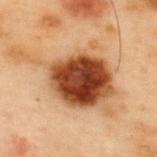Clinical impression:
Imaged during a routine full-body skin examination; the lesion was not biopsied and no histopathology is available.
Image and clinical context:
The lesion is located on the upper back. A 15 mm crop from a total-body photograph taken for skin-cancer surveillance. A male subject aged 53–57. Longest diameter approximately 11.5 mm. Automated image analysis of the tile measured a lesion area of about 38 mm², a shape eccentricity near 0.8, and a shape-asymmetry score of about 0.45 (0 = symmetric). The analysis additionally found a border-irregularity rating of about 6.5/10, a color-variation rating of about 9/10, and a peripheral color-asymmetry measure near 2.5.